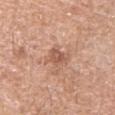* biopsy status — imaged on a skin check; not biopsied
* anatomic site — the left upper arm
* patient — male, in their mid-50s
* diameter — ~3 mm (longest diameter)
* automated metrics — a lesion area of about 5.5 mm² and two-axis asymmetry of about 0.25; a mean CIELAB color near L≈57 a*≈23 b*≈30, about 10 CIELAB-L* units darker than the surrounding skin, and a lesion-to-skin contrast of about 6.5 (normalized; higher = more distinct); a border-irregularity index near 3/10, internal color variation of about 3 on a 0–10 scale, and a peripheral color-asymmetry measure near 1; an automated nevus-likeness rating near 0 out of 100 and a lesion-detection confidence of about 100/100
* image — total-body-photography crop, ~15 mm field of view
* lighting — white-light